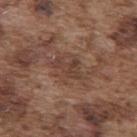Imaged during a routine full-body skin examination; the lesion was not biopsied and no histopathology is available. A male subject, aged 73–77. The lesion is on the upper back. A region of skin cropped from a whole-body photographic capture, roughly 15 mm wide.